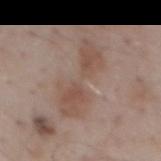Recorded during total-body skin imaging; not selected for excision or biopsy. A 15 mm crop from a total-body photograph taken for skin-cancer surveillance. From the back. The subject is a male approximately 55 years of age. Captured under white-light illumination.